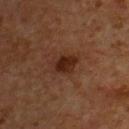<case>
<biopsy_status>not biopsied; imaged during a skin examination</biopsy_status>
<lighting>cross-polarized</lighting>
<image>
  <source>total-body photography crop</source>
  <field_of_view_mm>15</field_of_view_mm>
</image>
<site>chest</site>
<patient>
  <sex>male</sex>
  <age_approx>55</age_approx>
</patient>
<lesion_size>
  <long_diameter_mm_approx>3.5</long_diameter_mm_approx>
</lesion_size>
</case>